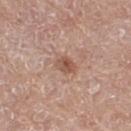Captured during whole-body skin photography for melanoma surveillance; the lesion was not biopsied.
A female patient roughly 75 years of age.
The lesion-visualizer software estimated an average lesion color of about L≈53 a*≈20 b*≈28 (CIELAB), about 10 CIELAB-L* units darker than the surrounding skin, and a lesion-to-skin contrast of about 7 (normalized; higher = more distinct).
This is a white-light tile.
From the right thigh.
About 2.5 mm across.
A roughly 15 mm field-of-view crop from a total-body skin photograph.